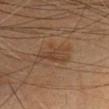Captured during whole-body skin photography for melanoma surveillance; the lesion was not biopsied.